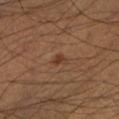<record>
<biopsy_status>not biopsied; imaged during a skin examination</biopsy_status>
<lighting>cross-polarized</lighting>
<site>left lower leg</site>
<lesion_size>
  <long_diameter_mm_approx>2.0</long_diameter_mm_approx>
</lesion_size>
<patient>
  <sex>male</sex>
  <age_approx>60</age_approx>
</patient>
<automated_metrics>
  <area_mm2_approx>2.0</area_mm2_approx>
  <eccentricity>0.7</eccentricity>
  <shape_asymmetry>0.35</shape_asymmetry>
  <cielab_L>36</cielab_L>
  <cielab_a>21</cielab_a>
  <cielab_b>30</cielab_b>
  <vs_skin_darker_L>8.0</vs_skin_darker_L>
  <vs_skin_contrast_norm>7.0</vs_skin_contrast_norm>
  <color_variation_0_10>0.5</color_variation_0_10>
  <peripheral_color_asymmetry>0.0</peripheral_color_asymmetry>
  <nevus_likeness_0_100>90</nevus_likeness_0_100>
</automated_metrics>
<image>
  <source>total-body photography crop</source>
  <field_of_view_mm>15</field_of_view_mm>
</image>
</record>